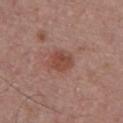Q: Is there a histopathology result?
A: total-body-photography surveillance lesion; no biopsy
Q: How was the tile lit?
A: white-light illumination
Q: Lesion size?
A: about 3 mm
Q: Where on the body is the lesion?
A: the chest
Q: What are the patient's age and sex?
A: male, aged 38 to 42
Q: What is the imaging modality?
A: total-body-photography crop, ~15 mm field of view